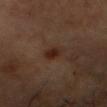Background:
The lesion is located on the head or neck. A male patient in their 50s. The tile uses cross-polarized illumination. A region of skin cropped from a whole-body photographic capture, roughly 15 mm wide. Approximately 2.5 mm at its widest.